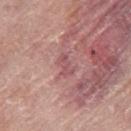lesion size=≈3 mm | location=the right thigh | subject=female, aged approximately 60 | acquisition=~15 mm crop, total-body skin-cancer survey | illumination=white-light.